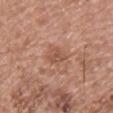{"biopsy_status": "not biopsied; imaged during a skin examination", "site": "chest", "image": {"source": "total-body photography crop", "field_of_view_mm": 15}, "patient": {"sex": "male", "age_approx": 65}, "automated_metrics": {"area_mm2_approx": 4.0, "eccentricity": 0.75, "vs_skin_darker_L": 8.0, "vs_skin_contrast_norm": 6.0}}